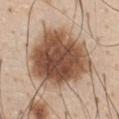Background:
On the front of the torso. Automated image analysis of the tile measured an average lesion color of about L≈50 a*≈19 b*≈30 (CIELAB), roughly 19 lightness units darker than nearby skin, and a lesion-to-skin contrast of about 12.5 (normalized; higher = more distinct). And it measured border irregularity of about 2.5 on a 0–10 scale, a within-lesion color-variation index near 6.5/10, and peripheral color asymmetry of about 2. The subject is a male approximately 60 years of age. Measured at roughly 8 mm in maximum diameter. A roughly 15 mm field-of-view crop from a total-body skin photograph. Imaged with white-light lighting.